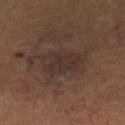The lesion was photographed on a routine skin check and not biopsied; there is no pathology result. A female subject, in their mid- to late 40s. Imaged with cross-polarized lighting. The lesion is located on the right leg. Cropped from a total-body skin-imaging series; the visible field is about 15 mm.